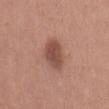• workup: imaged on a skin check; not biopsied
• lighting: white-light
• size: about 4.5 mm
• location: the back
• image: ~15 mm crop, total-body skin-cancer survey
• patient: female, about 35 years old